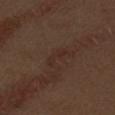Q: Lesion size?
A: ≈3.5 mm
Q: What is the anatomic site?
A: the arm
Q: What did automated image analysis measure?
A: a footprint of about 4 mm², an outline eccentricity of about 0.9 (0 = round, 1 = elongated), and a symmetry-axis asymmetry near 0.5; a mean CIELAB color near L≈28 a*≈16 b*≈22, a lesion–skin lightness drop of about 4, and a normalized border contrast of about 4.5; border irregularity of about 5.5 on a 0–10 scale, a color-variation rating of about 0/10, and peripheral color asymmetry of about 0
Q: Illumination type?
A: white-light
Q: Who is the patient?
A: male, about 70 years old
Q: How was this image acquired?
A: total-body-photography crop, ~15 mm field of view